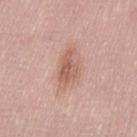Impression:
Captured during whole-body skin photography for melanoma surveillance; the lesion was not biopsied.
Context:
Automated image analysis of the tile measured an area of roughly 7.5 mm², an outline eccentricity of about 0.85 (0 = round, 1 = elongated), and two-axis asymmetry of about 0.35. It also reported lesion-presence confidence of about 100/100. The subject is a female approximately 30 years of age. This is a white-light tile. A 15 mm crop from a total-body photograph taken for skin-cancer surveillance. From the left thigh. Approximately 4.5 mm at its widest.